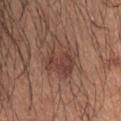Imaged during a routine full-body skin examination; the lesion was not biopsied and no histopathology is available. The patient is a male in their 30s. The tile uses white-light illumination. A 15 mm close-up extracted from a 3D total-body photography capture. The recorded lesion diameter is about 5 mm. On the head or neck.